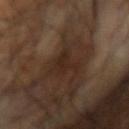Recorded during total-body skin imaging; not selected for excision or biopsy. On the left arm. The subject is a male aged 58–62. A 15 mm crop from a total-body photograph taken for skin-cancer surveillance. Imaged with cross-polarized lighting. The total-body-photography lesion software estimated a footprint of about 9 mm² and two-axis asymmetry of about 0.5. And it measured an automated nevus-likeness rating near 5 out of 100 and lesion-presence confidence of about 50/100.